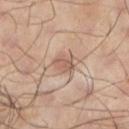Clinical impression: Recorded during total-body skin imaging; not selected for excision or biopsy. Clinical summary: A male patient, about 65 years old. The lesion is located on the left lower leg. A roughly 15 mm field-of-view crop from a total-body skin photograph. The tile uses cross-polarized illumination. Longest diameter approximately 3 mm.